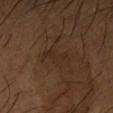The lesion was photographed on a routine skin check and not biopsied; there is no pathology result.
The subject is a male approximately 65 years of age.
The lesion is on the right forearm.
A roughly 15 mm field-of-view crop from a total-body skin photograph.